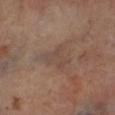  biopsy_status: not biopsied; imaged during a skin examination
  site: left lower leg
  patient:
    sex: male
    age_approx: 70
  image:
    source: total-body photography crop
    field_of_view_mm: 15